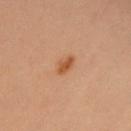Captured during whole-body skin photography for melanoma surveillance; the lesion was not biopsied. Captured under cross-polarized illumination. Cropped from a total-body skin-imaging series; the visible field is about 15 mm. A female patient aged 38 to 42. Located on the chest.